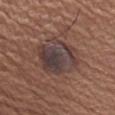Recorded during total-body skin imaging; not selected for excision or biopsy.
A roughly 15 mm field-of-view crop from a total-body skin photograph.
About 6 mm across.
The lesion is located on the left forearm.
A male patient aged 63 to 67.
Imaged with white-light lighting.